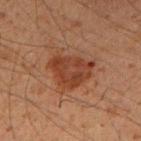Impression: Imaged during a routine full-body skin examination; the lesion was not biopsied and no histopathology is available. Acquisition and patient details: About 5 mm across. A male patient, aged 48 to 52. On the right upper arm. Imaged with cross-polarized lighting. Automated image analysis of the tile measured a footprint of about 14 mm², an outline eccentricity of about 0.65 (0 = round, 1 = elongated), and a symmetry-axis asymmetry near 0.35. The analysis additionally found internal color variation of about 3.5 on a 0–10 scale and peripheral color asymmetry of about 1. A 15 mm crop from a total-body photograph taken for skin-cancer surveillance.